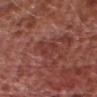patient = male, in their mid- to late 70s | anatomic site = the head or neck | size = about 3.5 mm | image = total-body-photography crop, ~15 mm field of view | tile lighting = white-light illumination.